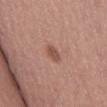The lesion was photographed on a routine skin check and not biopsied; there is no pathology result. The tile uses white-light illumination. Located on the right thigh. This image is a 15 mm lesion crop taken from a total-body photograph. A female patient roughly 50 years of age.